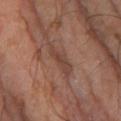Clinical impression: The lesion was tiled from a total-body skin photograph and was not biopsied. Background: A male patient roughly 65 years of age. Cropped from a whole-body photographic skin survey; the tile spans about 15 mm. Located on the left lower leg.